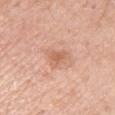| feature | finding |
|---|---|
| biopsy status | imaged on a skin check; not biopsied |
| anatomic site | the right upper arm |
| imaging modality | total-body-photography crop, ~15 mm field of view |
| tile lighting | white-light |
| subject | male, in their mid-50s |